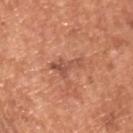Q: Is there a histopathology result?
A: no biopsy performed (imaged during a skin exam)
Q: How was this image acquired?
A: ~15 mm crop, total-body skin-cancer survey
Q: Automated lesion metrics?
A: an outline eccentricity of about 0.9 (0 = round, 1 = elongated) and a shape-asymmetry score of about 0.55 (0 = symmetric); a mean CIELAB color near L≈53 a*≈26 b*≈32 and a lesion-to-skin contrast of about 6 (normalized; higher = more distinct)
Q: How large is the lesion?
A: ≈4.5 mm
Q: Lesion location?
A: the upper back
Q: How was the tile lit?
A: white-light illumination
Q: Patient demographics?
A: male, about 65 years old